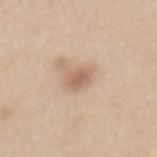Q: Was this lesion biopsied?
A: imaged on a skin check; not biopsied
Q: Where on the body is the lesion?
A: the mid back
Q: What is the imaging modality?
A: ~15 mm crop, total-body skin-cancer survey
Q: Who is the patient?
A: female, about 25 years old